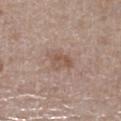Findings:
- biopsy status · catalogued during a skin exam; not biopsied
- lesion diameter · ≈4 mm
- location · the left lower leg
- patient · female, aged around 75
- image source · total-body-photography crop, ~15 mm field of view
- illumination · white-light